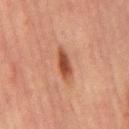Q: What did automated image analysis measure?
A: an average lesion color of about L≈39 a*≈23 b*≈28 (CIELAB), about 11 CIELAB-L* units darker than the surrounding skin, and a normalized lesion–skin contrast near 9.5; a within-lesion color-variation index near 3/10; an automated nevus-likeness rating near 100 out of 100 and lesion-presence confidence of about 100/100
Q: What kind of image is this?
A: total-body-photography crop, ~15 mm field of view
Q: What is the lesion's diameter?
A: ≈3.5 mm
Q: Illumination type?
A: cross-polarized
Q: Lesion location?
A: the abdomen
Q: Patient demographics?
A: male, about 65 years old